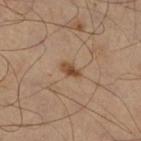follow-up: catalogued during a skin exam; not biopsied
patient: male, aged around 50
image source: ~15 mm crop, total-body skin-cancer survey
site: the left leg